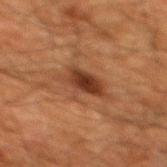Notes:
* biopsy status: imaged on a skin check; not biopsied
* image: ~15 mm tile from a whole-body skin photo
* subject: male, aged 58 to 62
* body site: the upper back